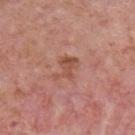Impression: Captured during whole-body skin photography for melanoma surveillance; the lesion was not biopsied. Acquisition and patient details: This is a white-light tile. A male patient, approximately 75 years of age. Located on the chest. A lesion tile, about 15 mm wide, cut from a 3D total-body photograph.